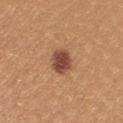Clinical impression:
This lesion was catalogued during total-body skin photography and was not selected for biopsy.
Context:
A lesion tile, about 15 mm wide, cut from a 3D total-body photograph. A female subject, in their mid- to late 20s. The lesion is on the left upper arm.